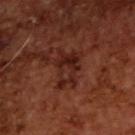This lesion was catalogued during total-body skin photography and was not selected for biopsy.
Automated image analysis of the tile measured about 7 CIELAB-L* units darker than the surrounding skin. And it measured internal color variation of about 6.5 on a 0–10 scale and a peripheral color-asymmetry measure near 2.5. The analysis additionally found an automated nevus-likeness rating near 5 out of 100 and a lesion-detection confidence of about 100/100.
A close-up tile cropped from a whole-body skin photograph, about 15 mm across.
The patient is a male aged 63–67.